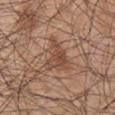Q: Was a biopsy performed?
A: no biopsy performed (imaged during a skin exam)
Q: What kind of image is this?
A: total-body-photography crop, ~15 mm field of view
Q: Who is the patient?
A: male, aged approximately 45
Q: Lesion location?
A: the right upper arm